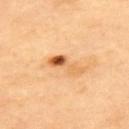Q: Was a biopsy performed?
A: total-body-photography surveillance lesion; no biopsy
Q: What is the lesion's diameter?
A: ≈4.5 mm
Q: Illumination type?
A: cross-polarized illumination
Q: What kind of image is this?
A: total-body-photography crop, ~15 mm field of view
Q: Lesion location?
A: the upper back